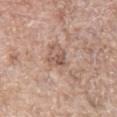Clinical impression:
Recorded during total-body skin imaging; not selected for excision or biopsy.
Image and clinical context:
The subject is a female in their 60s. The recorded lesion diameter is about 2.5 mm. Captured under white-light illumination. Located on the left thigh. The lesion-visualizer software estimated an average lesion color of about L≈55 a*≈19 b*≈25 (CIELAB) and roughly 10 lightness units darker than nearby skin. A close-up tile cropped from a whole-body skin photograph, about 15 mm across.